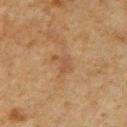biopsy_status: not biopsied; imaged during a skin examination
automated_metrics:
  eccentricity: 0.75
  shape_asymmetry: 0.4
  border_irregularity_0_10: 5.0
  color_variation_0_10: 1.0
patient:
  sex: male
  age_approx: 60
lighting: cross-polarized
site: chest
lesion_size:
  long_diameter_mm_approx: 2.5
image:
  source: total-body photography crop
  field_of_view_mm: 15